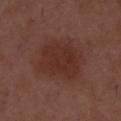{
  "biopsy_status": "not biopsied; imaged during a skin examination",
  "patient": {
    "sex": "male",
    "age_approx": 50
  },
  "lesion_size": {
    "long_diameter_mm_approx": 6.0
  },
  "automated_metrics": {
    "area_mm2_approx": 24.0,
    "eccentricity": 0.55,
    "shape_asymmetry": 0.2,
    "cielab_L": 31,
    "cielab_a": 21,
    "cielab_b": 23,
    "vs_skin_contrast_norm": 6.5
  },
  "image": {
    "source": "total-body photography crop",
    "field_of_view_mm": 15
  },
  "lighting": "white-light"
}